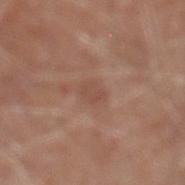Recorded during total-body skin imaging; not selected for excision or biopsy.
A close-up tile cropped from a whole-body skin photograph, about 15 mm across.
A male subject aged approximately 80.
Imaged with white-light lighting.
The lesion is located on the left lower leg.